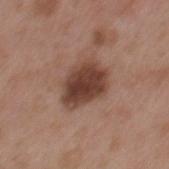Part of a total-body skin-imaging series; this lesion was reviewed on a skin check and was not flagged for biopsy.
A male patient approximately 55 years of age.
The recorded lesion diameter is about 5 mm.
Cropped from a whole-body photographic skin survey; the tile spans about 15 mm.
The lesion-visualizer software estimated an automated nevus-likeness rating near 80 out of 100 and a detector confidence of about 100 out of 100 that the crop contains a lesion.
The lesion is located on the mid back.